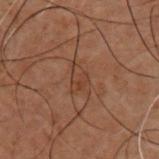<record>
  <biopsy_status>not biopsied; imaged during a skin examination</biopsy_status>
  <site>chest</site>
  <patient>
    <sex>male</sex>
    <age_approx>50</age_approx>
  </patient>
  <image>
    <source>total-body photography crop</source>
    <field_of_view_mm>15</field_of_view_mm>
  </image>
  <automated_metrics>
    <area_mm2_approx>5.0</area_mm2_approx>
    <eccentricity>0.8</eccentricity>
    <shape_asymmetry>0.4</shape_asymmetry>
    <cielab_L>30</cielab_L>
    <cielab_a>16</cielab_a>
    <cielab_b>23</cielab_b>
    <vs_skin_darker_L>5.0</vs_skin_darker_L>
    <vs_skin_contrast_norm>5.0</vs_skin_contrast_norm>
    <border_irregularity_0_10>4.5</border_irregularity_0_10>
  </automated_metrics>
</record>